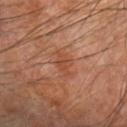Impression: This lesion was catalogued during total-body skin photography and was not selected for biopsy. Image and clinical context: A region of skin cropped from a whole-body photographic capture, roughly 15 mm wide. Located on the left forearm. The lesion's longest dimension is about 2.5 mm. The patient is a male in their 70s.